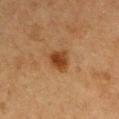Part of a total-body skin-imaging series; this lesion was reviewed on a skin check and was not flagged for biopsy. The patient is a female aged approximately 40. Automated image analysis of the tile measured an eccentricity of roughly 0.5 and two-axis asymmetry of about 0.3. The software also gave border irregularity of about 2.5 on a 0–10 scale, internal color variation of about 3.5 on a 0–10 scale, and a peripheral color-asymmetry measure near 1. From the left upper arm. Cropped from a total-body skin-imaging series; the visible field is about 15 mm. Imaged with cross-polarized lighting. The recorded lesion diameter is about 3 mm.